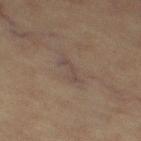The lesion was tiled from a total-body skin photograph and was not biopsied. About 3.5 mm across. Imaged with cross-polarized lighting. Automated image analysis of the tile measured a lesion area of about 3.5 mm² and an outline eccentricity of about 0.9 (0 = round, 1 = elongated). And it measured an average lesion color of about L≈37 a*≈12 b*≈18 (CIELAB), about 5 CIELAB-L* units darker than the surrounding skin, and a normalized lesion–skin contrast near 5. The analysis additionally found a lesion-detection confidence of about 50/100. A region of skin cropped from a whole-body photographic capture, roughly 15 mm wide. The lesion is located on the leg. The patient is a female aged 63 to 67.